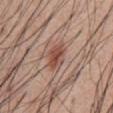Notes:
- biopsy status · catalogued during a skin exam; not biopsied
- image source · ~15 mm tile from a whole-body skin photo
- lighting · white-light illumination
- subject · male, in their mid-50s
- diameter · ~3.5 mm (longest diameter)
- body site · the abdomen
- automated lesion analysis · a footprint of about 6.5 mm², a shape eccentricity near 0.75, and a symmetry-axis asymmetry near 0.25; a lesion color around L≈50 a*≈22 b*≈27 in CIELAB, a lesion–skin lightness drop of about 11, and a normalized border contrast of about 8; border irregularity of about 2.5 on a 0–10 scale and peripheral color asymmetry of about 1.5; an automated nevus-likeness rating near 95 out of 100 and a detector confidence of about 100 out of 100 that the crop contains a lesion